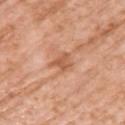Case summary:
– follow-up · total-body-photography surveillance lesion; no biopsy
– image · total-body-photography crop, ~15 mm field of view
– location · the upper back
– tile lighting · white-light illumination
– subject · female, in their 70s